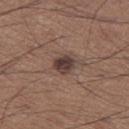Recorded during total-body skin imaging; not selected for excision or biopsy. The subject is a male aged 63–67. On the left thigh. Imaged with white-light lighting. This image is a 15 mm lesion crop taken from a total-body photograph.